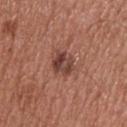Q: Was this lesion biopsied?
A: imaged on a skin check; not biopsied
Q: What is the anatomic site?
A: the upper back
Q: What are the patient's age and sex?
A: male, aged 53 to 57
Q: What is the imaging modality?
A: ~15 mm crop, total-body skin-cancer survey